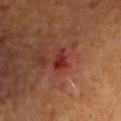Clinical impression: The lesion was photographed on a routine skin check and not biopsied; there is no pathology result. Background: A region of skin cropped from a whole-body photographic capture, roughly 15 mm wide. Located on the left upper arm. Captured under cross-polarized illumination. A male subject, about 55 years old.